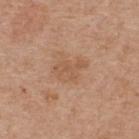Notes:
• imaging modality: total-body-photography crop, ~15 mm field of view
• anatomic site: the upper back
• patient: male, in their mid- to late 60s